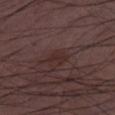Findings:
– image — 15 mm crop, total-body photography
– subject — male, aged 48 to 52
– site — the right thigh